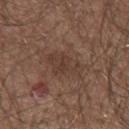| field | value |
|---|---|
| workup | catalogued during a skin exam; not biopsied |
| size | ≈4.5 mm |
| subject | male, about 75 years old |
| tile lighting | white-light illumination |
| image | ~15 mm tile from a whole-body skin photo |
| location | the left forearm |